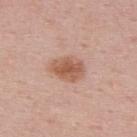workup = catalogued during a skin exam; not biopsied
body site = the upper back
diameter = ~4 mm (longest diameter)
patient = male, approximately 50 years of age
tile lighting = white-light illumination
acquisition = 15 mm crop, total-body photography